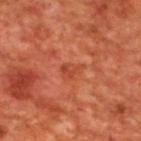Impression: The lesion was photographed on a routine skin check and not biopsied; there is no pathology result. Clinical summary: An algorithmic analysis of the crop reported two-axis asymmetry of about 0.45. And it measured about 7 CIELAB-L* units darker than the surrounding skin and a normalized border contrast of about 5.5. It also reported a border-irregularity index near 4/10 and a within-lesion color-variation index near 2.5/10. A close-up tile cropped from a whole-body skin photograph, about 15 mm across. On the upper back. The tile uses cross-polarized illumination. The subject is a male approximately 70 years of age. Measured at roughly 2.5 mm in maximum diameter.